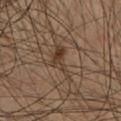Q: Is there a histopathology result?
A: no biopsy performed (imaged during a skin exam)
Q: Automated lesion metrics?
A: a lesion area of about 6 mm², an outline eccentricity of about 0.9 (0 = round, 1 = elongated), and a symmetry-axis asymmetry near 0.6; a color-variation rating of about 4/10 and a peripheral color-asymmetry measure near 1; a lesion-detection confidence of about 75/100
Q: How large is the lesion?
A: ~5 mm (longest diameter)
Q: Illumination type?
A: cross-polarized
Q: Lesion location?
A: the chest
Q: Who is the patient?
A: male, aged 58–62
Q: What kind of image is this?
A: 15 mm crop, total-body photography